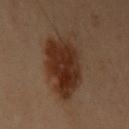notes: imaged on a skin check; not biopsied
lighting: cross-polarized illumination
lesion diameter: ~7 mm (longest diameter)
image: ~15 mm crop, total-body skin-cancer survey
patient: female, about 40 years old
anatomic site: the left upper arm